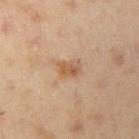No biopsy was performed on this lesion — it was imaged during a full skin examination and was not determined to be concerning. A male subject, aged around 55. Imaged with cross-polarized lighting. The lesion is on the right upper arm. A 15 mm crop from a total-body photograph taken for skin-cancer surveillance.